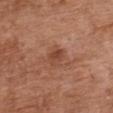<lesion>
<biopsy_status>not biopsied; imaged during a skin examination</biopsy_status>
<lighting>white-light</lighting>
<site>chest</site>
<automated_metrics>
  <area_mm2_approx>4.0</area_mm2_approx>
  <eccentricity>0.65</eccentricity>
  <shape_asymmetry>0.3</shape_asymmetry>
  <border_irregularity_0_10>2.5</border_irregularity_0_10>
  <color_variation_0_10>3.0</color_variation_0_10>
  <peripheral_color_asymmetry>1.0</peripheral_color_asymmetry>
  <nevus_likeness_0_100>25</nevus_likeness_0_100>
  <lesion_detection_confidence_0_100>100</lesion_detection_confidence_0_100>
</automated_metrics>
<image>
  <source>total-body photography crop</source>
  <field_of_view_mm>15</field_of_view_mm>
</image>
<lesion_size>
  <long_diameter_mm_approx>2.5</long_diameter_mm_approx>
</lesion_size>
<patient>
  <sex>female</sex>
  <age_approx>65</age_approx>
</patient>
</lesion>